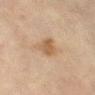notes: total-body-photography surveillance lesion; no biopsy
anatomic site: the abdomen
tile lighting: cross-polarized illumination
imaging modality: total-body-photography crop, ~15 mm field of view
patient: female, in their 80s
size: ~3 mm (longest diameter)